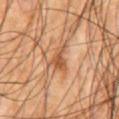Clinical impression:
The lesion was photographed on a routine skin check and not biopsied; there is no pathology result.
Image and clinical context:
A 15 mm close-up tile from a total-body photography series done for melanoma screening. This is a cross-polarized tile. A male subject, in their mid- to late 60s. On the chest. The lesion's longest dimension is about 3 mm.